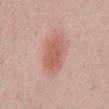Findings:
* workup: no biopsy performed (imaged during a skin exam)
* body site: the chest
* subject: male, aged around 60
* lesion size: ~5.5 mm (longest diameter)
* image source: 15 mm crop, total-body photography
* lighting: white-light illumination